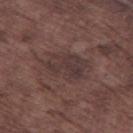Part of a total-body skin-imaging series; this lesion was reviewed on a skin check and was not flagged for biopsy. The lesion-visualizer software estimated a footprint of about 13 mm² and an eccentricity of roughly 0.8. And it measured a lesion–skin lightness drop of about 6 and a normalized border contrast of about 6.5. The analysis additionally found internal color variation of about 3.5 on a 0–10 scale. Cropped from a whole-body photographic skin survey; the tile spans about 15 mm. Measured at roughly 5.5 mm in maximum diameter. The tile uses white-light illumination. On the left thigh. The subject is a male approximately 75 years of age.